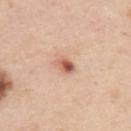Notes:
- workup · catalogued during a skin exam; not biopsied
- acquisition · total-body-photography crop, ~15 mm field of view
- patient · male, approximately 45 years of age
- automated metrics · an outline eccentricity of about 0.75 (0 = round, 1 = elongated) and a symmetry-axis asymmetry near 0.3; an average lesion color of about L≈60 a*≈23 b*≈32 (CIELAB), roughly 14 lightness units darker than nearby skin, and a normalized lesion–skin contrast near 9
- body site · the upper back
- lighting · white-light illumination